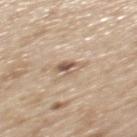A lesion tile, about 15 mm wide, cut from a 3D total-body photograph. Captured under white-light illumination. From the mid back. The patient is a male aged approximately 70.